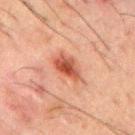No biopsy was performed on this lesion — it was imaged during a full skin examination and was not determined to be concerning. Captured under cross-polarized illumination. The total-body-photography lesion software estimated a footprint of about 6.5 mm² and two-axis asymmetry of about 0.2. It also reported an average lesion color of about L≈42 a*≈25 b*≈28 (CIELAB) and a lesion–skin lightness drop of about 11. It also reported a border-irregularity index near 2/10. The analysis additionally found a nevus-likeness score of about 95/100 and a lesion-detection confidence of about 100/100. Cropped from a whole-body photographic skin survey; the tile spans about 15 mm. Measured at roughly 4 mm in maximum diameter. The lesion is on the mid back. A male patient, about 50 years old.